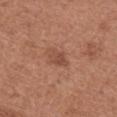Captured during whole-body skin photography for melanoma surveillance; the lesion was not biopsied. A female patient, aged 48 to 52. This is a white-light tile. On the chest. Measured at roughly 3 mm in maximum diameter. A 15 mm crop from a total-body photograph taken for skin-cancer surveillance.